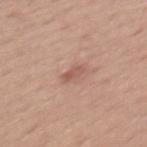Findings:
– follow-up: catalogued during a skin exam; not biopsied
– body site: the back
– lesion diameter: about 2.5 mm
– lighting: white-light illumination
– patient: male, aged 48–52
– image source: total-body-photography crop, ~15 mm field of view